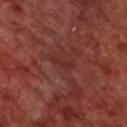Recorded during total-body skin imaging; not selected for excision or biopsy. Imaged with cross-polarized lighting. A male patient, aged 68–72. The lesion's longest dimension is about 3 mm. A close-up tile cropped from a whole-body skin photograph, about 15 mm across. From the upper back.